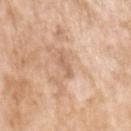Assessment:
The lesion was tiled from a total-body skin photograph and was not biopsied.
Background:
Captured under white-light illumination. The lesion is on the left upper arm. About 2.5 mm across. A female subject, about 75 years old. An algorithmic analysis of the crop reported an area of roughly 2.5 mm² and a shape-asymmetry score of about 0.35 (0 = symmetric). And it measured a border-irregularity rating of about 4/10 and a peripheral color-asymmetry measure near 0. It also reported an automated nevus-likeness rating near 0 out of 100 and lesion-presence confidence of about 100/100. A 15 mm close-up tile from a total-body photography series done for melanoma screening.